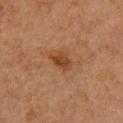Assessment:
This lesion was catalogued during total-body skin photography and was not selected for biopsy.
Image and clinical context:
The lesion is located on the chest. Automated tile analysis of the lesion measured an average lesion color of about L≈36 a*≈21 b*≈32 (CIELAB) and a lesion–skin lightness drop of about 8. The analysis additionally found a lesion-detection confidence of about 100/100. Measured at roughly 3 mm in maximum diameter. Imaged with cross-polarized lighting. A female patient, roughly 40 years of age. Cropped from a total-body skin-imaging series; the visible field is about 15 mm.